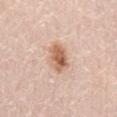<lesion>
<patient>
  <sex>male</sex>
  <age_approx>80</age_approx>
</patient>
<image>
  <source>total-body photography crop</source>
  <field_of_view_mm>15</field_of_view_mm>
</image>
<lesion_size>
  <long_diameter_mm_approx>3.5</long_diameter_mm_approx>
</lesion_size>
<site>lower back</site>
<lighting>white-light</lighting>
</lesion>